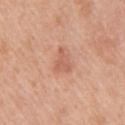Notes:
• illumination: white-light illumination
• site: the arm
• imaging modality: ~15 mm crop, total-body skin-cancer survey
• patient: female, aged 38–42